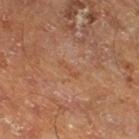{
  "biopsy_status": "not biopsied; imaged during a skin examination",
  "image": {
    "source": "total-body photography crop",
    "field_of_view_mm": 15
  },
  "lighting": "cross-polarized",
  "automated_metrics": {
    "cielab_L": 47,
    "cielab_a": 22,
    "cielab_b": 33
  },
  "patient": {
    "sex": "male",
    "age_approx": 65
  },
  "site": "leg"
}